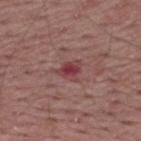Q: Was this lesion biopsied?
A: total-body-photography surveillance lesion; no biopsy
Q: Lesion size?
A: ~2.5 mm (longest diameter)
Q: Where on the body is the lesion?
A: the upper back
Q: What kind of image is this?
A: ~15 mm crop, total-body skin-cancer survey
Q: What did automated image analysis measure?
A: a mean CIELAB color near L≈41 a*≈27 b*≈19 and a normalized lesion–skin contrast near 8
Q: How was the tile lit?
A: white-light illumination
Q: Who is the patient?
A: male, in their mid-50s